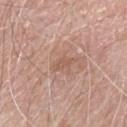Impression:
This lesion was catalogued during total-body skin photography and was not selected for biopsy.
Context:
A roughly 15 mm field-of-view crop from a total-body skin photograph. The lesion is on the chest. The lesion's longest dimension is about 3 mm. An algorithmic analysis of the crop reported a border-irregularity rating of about 4/10 and peripheral color asymmetry of about 0.5. A male subject, aged 63 to 67.